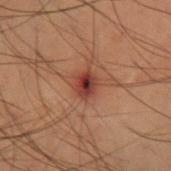The lesion was tiled from a total-body skin photograph and was not biopsied.
A roughly 15 mm field-of-view crop from a total-body skin photograph.
The patient is a male in their mid-50s.
The lesion is located on the right forearm.